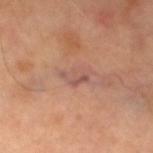patient: female, approximately 60 years of age
illumination: cross-polarized
lesion diameter: ≈3.5 mm
automated lesion analysis: a mean CIELAB color near L≈55 a*≈22 b*≈26 and a normalized border contrast of about 6; a border-irregularity rating of about 4/10 and internal color variation of about 3.5 on a 0–10 scale; a classifier nevus-likeness of about 0/100 and a lesion-detection confidence of about 70/100
site: the right leg
image: ~15 mm crop, total-body skin-cancer survey